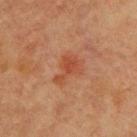biopsy status: imaged on a skin check; not biopsied | patient: male, about 60 years old | size: about 4 mm | site: the upper back | imaging modality: ~15 mm crop, total-body skin-cancer survey.